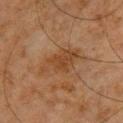Q: Was a biopsy performed?
A: total-body-photography surveillance lesion; no biopsy
Q: Patient demographics?
A: male, in their 60s
Q: Lesion location?
A: the chest
Q: What is the imaging modality?
A: ~15 mm crop, total-body skin-cancer survey
Q: What lighting was used for the tile?
A: cross-polarized illumination
Q: What is the lesion's diameter?
A: ≈5.5 mm